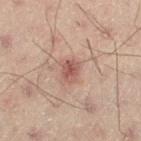Clinical impression:
The lesion was tiled from a total-body skin photograph and was not biopsied.
Background:
Imaged with cross-polarized lighting. The patient is a male aged 48 to 52. About 2.5 mm across. The lesion is on the leg. A lesion tile, about 15 mm wide, cut from a 3D total-body photograph.